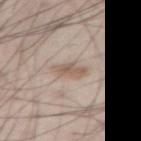Acquisition and patient details: The lesion-visualizer software estimated a mean CIELAB color near L≈58 a*≈14 b*≈26, a lesion–skin lightness drop of about 9, and a normalized border contrast of about 7. The patient is a male approximately 30 years of age. From the front of the torso. A region of skin cropped from a whole-body photographic capture, roughly 15 mm wide.